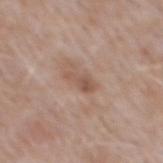| key | value |
|---|---|
| notes | total-body-photography surveillance lesion; no biopsy |
| subject | male, about 50 years old |
| image source | total-body-photography crop, ~15 mm field of view |
| illumination | white-light illumination |
| location | the mid back |
| TBP lesion metrics | a footprint of about 4 mm², an outline eccentricity of about 0.85 (0 = round, 1 = elongated), and a shape-asymmetry score of about 0.35 (0 = symmetric); a border-irregularity index near 3.5/10 and peripheral color asymmetry of about 0.5; a nevus-likeness score of about 0/100 and a detector confidence of about 100 out of 100 that the crop contains a lesion |